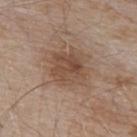Assessment:
Imaged during a routine full-body skin examination; the lesion was not biopsied and no histopathology is available.
Image and clinical context:
The tile uses white-light illumination. On the upper back. Cropped from a total-body skin-imaging series; the visible field is about 15 mm. An algorithmic analysis of the crop reported a mean CIELAB color near L≈48 a*≈17 b*≈28 and roughly 8 lightness units darker than nearby skin. It also reported a border-irregularity index near 2/10 and peripheral color asymmetry of about 2. The software also gave an automated nevus-likeness rating near 55 out of 100 and lesion-presence confidence of about 100/100. A male subject about 55 years old.